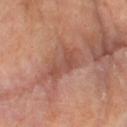Impression: Imaged during a routine full-body skin examination; the lesion was not biopsied and no histopathology is available.